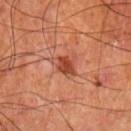Q: What is the anatomic site?
A: the arm
Q: How large is the lesion?
A: about 2.5 mm
Q: How was this image acquired?
A: 15 mm crop, total-body photography
Q: Automated lesion metrics?
A: an area of roughly 5 mm², a shape eccentricity near 0.65, and a shape-asymmetry score of about 0.25 (0 = symmetric); a mean CIELAB color near L≈47 a*≈31 b*≈35, about 12 CIELAB-L* units darker than the surrounding skin, and a normalized border contrast of about 8.5; a border-irregularity index near 3/10, a within-lesion color-variation index near 3.5/10, and radial color variation of about 1; an automated nevus-likeness rating near 80 out of 100 and a lesion-detection confidence of about 100/100
Q: How was the tile lit?
A: cross-polarized illumination
Q: Who is the patient?
A: male, in their mid-60s